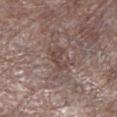workup: total-body-photography surveillance lesion; no biopsy
diameter: ~3.5 mm (longest diameter)
image source: total-body-photography crop, ~15 mm field of view
anatomic site: the left lower leg
subject: male, aged approximately 70